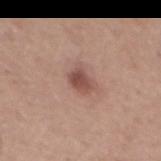{"biopsy_status": "not biopsied; imaged during a skin examination", "site": "mid back", "image": {"source": "total-body photography crop", "field_of_view_mm": 15}, "patient": {"sex": "male", "age_approx": 55}, "automated_metrics": {"area_mm2_approx": 4.5, "eccentricity": 0.7, "shape_asymmetry": 0.25}, "lighting": "white-light"}